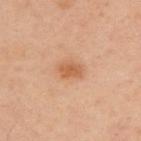No biopsy was performed on this lesion — it was imaged during a full skin examination and was not determined to be concerning. Longest diameter approximately 3 mm. The total-body-photography lesion software estimated a lesion area of about 4.5 mm², an eccentricity of roughly 0.75, and a symmetry-axis asymmetry near 0.2. And it measured a mean CIELAB color near L≈58 a*≈24 b*≈36, about 9 CIELAB-L* units darker than the surrounding skin, and a normalized border contrast of about 7. It also reported a border-irregularity index near 2/10, internal color variation of about 1.5 on a 0–10 scale, and radial color variation of about 0.5. The software also gave a classifier nevus-likeness of about 70/100 and lesion-presence confidence of about 100/100. Captured under cross-polarized illumination. From the upper back. A male subject, aged 43–47. This image is a 15 mm lesion crop taken from a total-body photograph.